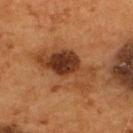Q: Was a biopsy performed?
A: no biopsy performed (imaged during a skin exam)
Q: What is the anatomic site?
A: the upper back
Q: Illumination type?
A: cross-polarized illumination
Q: How large is the lesion?
A: ~7.5 mm (longest diameter)
Q: What kind of image is this?
A: 15 mm crop, total-body photography
Q: What are the patient's age and sex?
A: male, aged 53 to 57